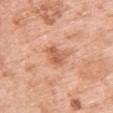The lesion was photographed on a routine skin check and not biopsied; there is no pathology result. From the chest. Measured at roughly 3 mm in maximum diameter. A female subject, aged around 50. Imaged with white-light lighting. This image is a 15 mm lesion crop taken from a total-body photograph.